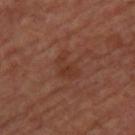| key | value |
|---|---|
| notes | imaged on a skin check; not biopsied |
| patient | female, roughly 45 years of age |
| image source | 15 mm crop, total-body photography |
| body site | the upper back |